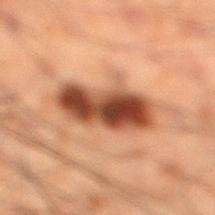Assessment:
No biopsy was performed on this lesion — it was imaged during a full skin examination and was not determined to be concerning.
Background:
A 15 mm close-up extracted from a 3D total-body photography capture. Automated image analysis of the tile measured an area of roughly 20 mm², an outline eccentricity of about 0.9 (0 = round, 1 = elongated), and a symmetry-axis asymmetry near 0.15. Imaged with cross-polarized lighting. The lesion is on the leg. The recorded lesion diameter is about 7.5 mm. A male patient aged around 50.